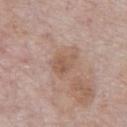biopsy status: catalogued during a skin exam; not biopsied
illumination: white-light
subject: male, aged 68–72
diameter: ~3 mm (longest diameter)
anatomic site: the chest
image source: ~15 mm crop, total-body skin-cancer survey
TBP lesion metrics: a mean CIELAB color near L≈55 a*≈19 b*≈28, roughly 7 lightness units darker than nearby skin, and a lesion-to-skin contrast of about 5.5 (normalized; higher = more distinct)